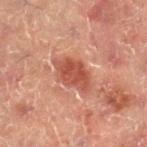  biopsy_status: not biopsied; imaged during a skin examination
  patient:
    sex: male
    age_approx: 50
  automated_metrics:
    area_mm2_approx: 10.0
    eccentricity: 0.8
    shape_asymmetry: 0.2
    color_variation_0_10: 2.5
    peripheral_color_asymmetry: 1.0
    nevus_likeness_0_100: 65
    lesion_detection_confidence_0_100: 100
  image:
    source: total-body photography crop
    field_of_view_mm: 15
  site: right lower leg
  lighting: cross-polarized
  lesion_size:
    long_diameter_mm_approx: 5.0